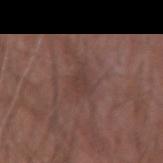<record>
  <biopsy_status>not biopsied; imaged during a skin examination</biopsy_status>
  <lesion_size>
    <long_diameter_mm_approx>3.0</long_diameter_mm_approx>
  </lesion_size>
  <patient>
    <sex>male</sex>
    <age_approx>55</age_approx>
  </patient>
  <site>arm</site>
  <image>
    <source>total-body photography crop</source>
    <field_of_view_mm>15</field_of_view_mm>
  </image>
  <lighting>white-light</lighting>
  <automated_metrics>
    <area_mm2_approx>4.5</area_mm2_approx>
    <shape_asymmetry>0.45</shape_asymmetry>
    <cielab_L>38</cielab_L>
    <cielab_a>17</cielab_a>
    <cielab_b>21</cielab_b>
    <vs_skin_darker_L>5.0</vs_skin_darker_L>
    <vs_skin_contrast_norm>4.5</vs_skin_contrast_norm>
    <border_irregularity_0_10>4.5</border_irregularity_0_10>
    <color_variation_0_10>1.5</color_variation_0_10>
  </automated_metrics>
</record>